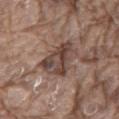Recorded during total-body skin imaging; not selected for excision or biopsy. The tile uses white-light illumination. A male patient, about 80 years old. The recorded lesion diameter is about 5 mm. Cropped from a whole-body photographic skin survey; the tile spans about 15 mm. The lesion is located on the mid back.